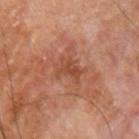Clinical impression:
No biopsy was performed on this lesion — it was imaged during a full skin examination and was not determined to be concerning.
Background:
From the left upper arm. A male patient, roughly 70 years of age. A close-up tile cropped from a whole-body skin photograph, about 15 mm across.